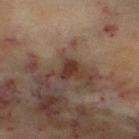{"biopsy_status": "not biopsied; imaged during a skin examination", "site": "left thigh", "image": {"source": "total-body photography crop", "field_of_view_mm": 15}, "lighting": "cross-polarized", "patient": {"sex": "female", "age_approx": 60}, "lesion_size": {"long_diameter_mm_approx": 2.5}, "automated_metrics": {"nevus_likeness_0_100": 0, "lesion_detection_confidence_0_100": 100}}